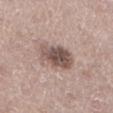Captured under white-light illumination. Located on the left lower leg. A female subject aged 48–52. The recorded lesion diameter is about 4.5 mm. A roughly 15 mm field-of-view crop from a total-body skin photograph. Automated tile analysis of the lesion measured a footprint of about 11 mm². The software also gave a mean CIELAB color near L≈52 a*≈16 b*≈21. The software also gave a border-irregularity rating of about 2.5/10 and a within-lesion color-variation index near 5.5/10. The analysis additionally found lesion-presence confidence of about 100/100.